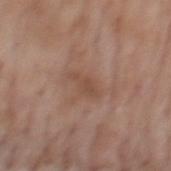This lesion was catalogued during total-body skin photography and was not selected for biopsy. A lesion tile, about 15 mm wide, cut from a 3D total-body photograph. The patient is a male about 75 years old. On the mid back. Approximately 3.5 mm at its widest.